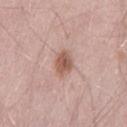Impression:
This lesion was catalogued during total-body skin photography and was not selected for biopsy.
Clinical summary:
A lesion tile, about 15 mm wide, cut from a 3D total-body photograph. The tile uses white-light illumination. On the leg. A male subject, roughly 50 years of age. Measured at roughly 3 mm in maximum diameter.